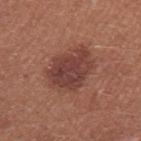Clinical impression: Recorded during total-body skin imaging; not selected for excision or biopsy. Background: A roughly 15 mm field-of-view crop from a total-body skin photograph. On the left upper arm. Longest diameter approximately 5.5 mm. Captured under white-light illumination. A female subject, aged 23 to 27.